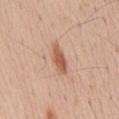The lesion was tiled from a total-body skin photograph and was not biopsied.
The tile uses white-light illumination.
The recorded lesion diameter is about 4.5 mm.
Cropped from a total-body skin-imaging series; the visible field is about 15 mm.
A male patient, about 55 years old.
The lesion-visualizer software estimated a footprint of about 6.5 mm² and a shape-asymmetry score of about 0.2 (0 = symmetric). It also reported a mean CIELAB color near L≈59 a*≈22 b*≈32 and a normalized border contrast of about 8. And it measured a border-irregularity index near 2.5/10 and internal color variation of about 3 on a 0–10 scale.
The lesion is located on the abdomen.